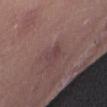Assessment:
Imaged during a routine full-body skin examination; the lesion was not biopsied and no histopathology is available.
Image and clinical context:
The patient is a female about 45 years old. A lesion tile, about 15 mm wide, cut from a 3D total-body photograph. About 2.5 mm across. Automated image analysis of the tile measured a border-irregularity rating of about 4/10 and a peripheral color-asymmetry measure near 0. It also reported an automated nevus-likeness rating near 0 out of 100 and a detector confidence of about 65 out of 100 that the crop contains a lesion. From the abdomen.